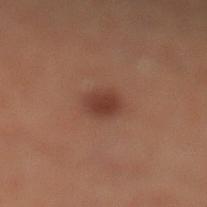Q: What kind of image is this?
A: ~15 mm tile from a whole-body skin photo
Q: Lesion size?
A: ~2.5 mm (longest diameter)
Q: Illumination type?
A: cross-polarized
Q: Automated lesion metrics?
A: a shape eccentricity near 0.25 and two-axis asymmetry of about 0.1; a lesion color around L≈28 a*≈18 b*≈21 in CIELAB, roughly 7 lightness units darker than nearby skin, and a normalized lesion–skin contrast near 7.5; border irregularity of about 1 on a 0–10 scale, a color-variation rating of about 1.5/10, and radial color variation of about 0.5; lesion-presence confidence of about 100/100
Q: Where on the body is the lesion?
A: the left lower leg
Q: Who is the patient?
A: male, aged 58 to 62